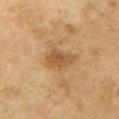Clinical impression: The lesion was photographed on a routine skin check and not biopsied; there is no pathology result. Image and clinical context: Imaged with cross-polarized lighting. A roughly 15 mm field-of-view crop from a total-body skin photograph. Measured at roughly 3.5 mm in maximum diameter. The lesion is on the left upper arm. The patient is a female aged approximately 60. The lesion-visualizer software estimated an average lesion color of about L≈47 a*≈17 b*≈34 (CIELAB) and a lesion–skin lightness drop of about 9. The software also gave a within-lesion color-variation index near 2.5/10 and peripheral color asymmetry of about 1. The software also gave a nevus-likeness score of about 50/100 and lesion-presence confidence of about 100/100.